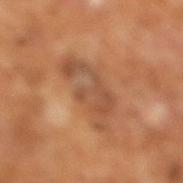Recorded during total-body skin imaging; not selected for excision or biopsy.
The recorded lesion diameter is about 6.5 mm.
The subject is a male aged 63–67.
Imaged with cross-polarized lighting.
A region of skin cropped from a whole-body photographic capture, roughly 15 mm wide.